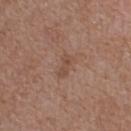No biopsy was performed on this lesion — it was imaged during a full skin examination and was not determined to be concerning.
Captured under white-light illumination.
On the upper back.
Cropped from a whole-body photographic skin survey; the tile spans about 15 mm.
A female subject, approximately 40 years of age.
The lesion-visualizer software estimated an area of roughly 4 mm², an outline eccentricity of about 0.85 (0 = round, 1 = elongated), and a symmetry-axis asymmetry near 0.3. The analysis additionally found a lesion–skin lightness drop of about 7 and a lesion-to-skin contrast of about 5 (normalized; higher = more distinct). The software also gave a peripheral color-asymmetry measure near 0.5.
Approximately 3 mm at its widest.